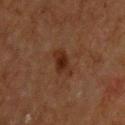Assessment: Recorded during total-body skin imaging; not selected for excision or biopsy. Acquisition and patient details: The tile uses cross-polarized illumination. A male patient, aged 63 to 67. Longest diameter approximately 3.5 mm. This image is a 15 mm lesion crop taken from a total-body photograph. The lesion is located on the upper back.